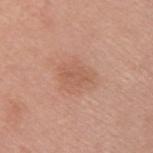Part of a total-body skin-imaging series; this lesion was reviewed on a skin check and was not flagged for biopsy.